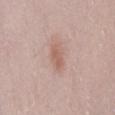Clinical impression:
Recorded during total-body skin imaging; not selected for excision or biopsy.
Clinical summary:
The lesion is on the mid back. A 15 mm close-up tile from a total-body photography series done for melanoma screening. A female patient aged around 50.